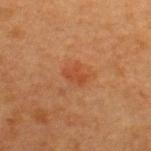notes: imaged on a skin check; not biopsied | lesion size: ≈2.5 mm | image source: 15 mm crop, total-body photography | subject: female, approximately 40 years of age | anatomic site: the upper back | lighting: cross-polarized | TBP lesion metrics: an area of roughly 3.5 mm², an eccentricity of roughly 0.7, and two-axis asymmetry of about 0.35; a lesion color around L≈41 a*≈26 b*≈34 in CIELAB and a lesion–skin lightness drop of about 6.